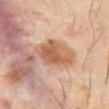Measured at roughly 4.5 mm in maximum diameter. The lesion is located on the abdomen. Captured under cross-polarized illumination. A male patient aged 58–62. A close-up tile cropped from a whole-body skin photograph, about 15 mm across.